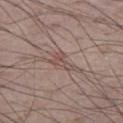Assessment:
Part of a total-body skin-imaging series; this lesion was reviewed on a skin check and was not flagged for biopsy.
Acquisition and patient details:
Longest diameter approximately 3 mm. The lesion is on the right thigh. A 15 mm close-up tile from a total-body photography series done for melanoma screening. The patient is a male aged 73–77. Captured under white-light illumination. Automated image analysis of the tile measured an area of roughly 3.5 mm², an outline eccentricity of about 0.8 (0 = round, 1 = elongated), and a symmetry-axis asymmetry near 0.6. The analysis additionally found a mean CIELAB color near L≈50 a*≈17 b*≈21, about 8 CIELAB-L* units darker than the surrounding skin, and a normalized lesion–skin contrast near 6. It also reported a classifier nevus-likeness of about 0/100 and lesion-presence confidence of about 80/100.